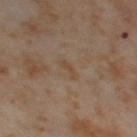biopsy status=imaged on a skin check; not biopsied | site=the right thigh | diameter=about 2.5 mm | tile lighting=cross-polarized | acquisition=~15 mm crop, total-body skin-cancer survey | patient=female, aged 53 to 57.